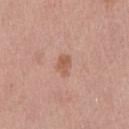{"lighting": "white-light", "site": "chest", "patient": {"sex": "male", "age_approx": 25}, "automated_metrics": {"area_mm2_approx": 3.5, "shape_asymmetry": 0.25, "cielab_L": 57, "cielab_a": 22, "cielab_b": 30, "vs_skin_darker_L": 9.0, "border_irregularity_0_10": 2.0, "color_variation_0_10": 2.0, "peripheral_color_asymmetry": 0.5, "nevus_likeness_0_100": 10, "lesion_detection_confidence_0_100": 100}, "image": {"source": "total-body photography crop", "field_of_view_mm": 15}, "lesion_size": {"long_diameter_mm_approx": 2.5}}